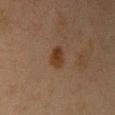The lesion was photographed on a routine skin check and not biopsied; there is no pathology result.